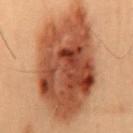<tbp_lesion>
  <biopsy_status>not biopsied; imaged during a skin examination</biopsy_status>
  <site>back</site>
  <lighting>cross-polarized</lighting>
  <automated_metrics>
    <area_mm2_approx>75.0</area_mm2_approx>
    <eccentricity>0.8</eccentricity>
    <border_irregularity_0_10>2.0</border_irregularity_0_10>
    <color_variation_0_10>8.0</color_variation_0_10>
    <peripheral_color_asymmetry>3.0</peripheral_color_asymmetry>
  </automated_metrics>
  <image>
    <source>total-body photography crop</source>
    <field_of_view_mm>15</field_of_view_mm>
  </image>
  <patient>
    <sex>male</sex>
    <age_approx>55</age_approx>
  </patient>
  <lesion_size>
    <long_diameter_mm_approx>13.0</long_diameter_mm_approx>
  </lesion_size>
</tbp_lesion>